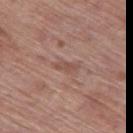Assessment: Part of a total-body skin-imaging series; this lesion was reviewed on a skin check and was not flagged for biopsy. Acquisition and patient details: A close-up tile cropped from a whole-body skin photograph, about 15 mm across. From the leg. A male subject aged around 70.